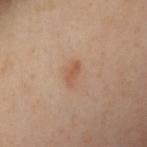Assessment: Imaged during a routine full-body skin examination; the lesion was not biopsied and no histopathology is available. Background: On the left arm. A female subject, aged around 40. About 2.5 mm across. The total-body-photography lesion software estimated an area of roughly 2.5 mm² and two-axis asymmetry of about 0.25. The software also gave an average lesion color of about L≈56 a*≈21 b*≈33 (CIELAB), a lesion–skin lightness drop of about 8, and a normalized border contrast of about 6.5. A region of skin cropped from a whole-body photographic capture, roughly 15 mm wide.